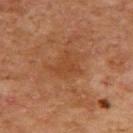imaging modality: 15 mm crop, total-body photography
body site: the upper back
tile lighting: cross-polarized
patient: female, roughly 60 years of age